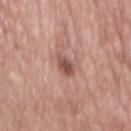The recorded lesion diameter is about 4 mm. A female subject, aged approximately 75. Captured under white-light illumination. A 15 mm close-up tile from a total-body photography series done for melanoma screening. On the back.